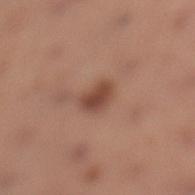Part of a total-body skin-imaging series; this lesion was reviewed on a skin check and was not flagged for biopsy.